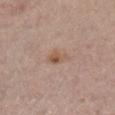  biopsy_status: not biopsied; imaged during a skin examination
  image:
    source: total-body photography crop
    field_of_view_mm: 15
  lesion_size:
    long_diameter_mm_approx: 3.0
  site: right lower leg
  patient:
    sex: female
    age_approx: 65
  lighting: white-light